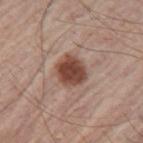Impression:
Captured during whole-body skin photography for melanoma surveillance; the lesion was not biopsied.
Context:
The tile uses white-light illumination. Cropped from a total-body skin-imaging series; the visible field is about 15 mm. A male subject, in their mid-60s. The lesion is located on the left upper arm. About 3.5 mm across.